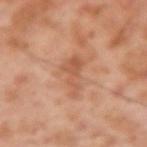Captured during whole-body skin photography for melanoma surveillance; the lesion was not biopsied.
The lesion is located on the left upper arm.
About 5 mm across.
A region of skin cropped from a whole-body photographic capture, roughly 15 mm wide.
A male subject aged approximately 55.
Captured under cross-polarized illumination.